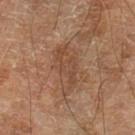Imaged during a routine full-body skin examination; the lesion was not biopsied and no histopathology is available. A roughly 15 mm field-of-view crop from a total-body skin photograph. From the left lower leg. A male patient, aged around 70.